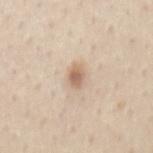Clinical impression:
Part of a total-body skin-imaging series; this lesion was reviewed on a skin check and was not flagged for biopsy.
Acquisition and patient details:
Approximately 2.5 mm at its widest. The lesion is located on the mid back. A female subject roughly 45 years of age. A 15 mm crop from a total-body photograph taken for skin-cancer surveillance.